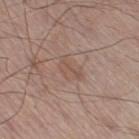follow-up: no biopsy performed (imaged during a skin exam) | anatomic site: the right thigh | patient: male, aged around 55 | image: ~15 mm tile from a whole-body skin photo | tile lighting: white-light | size: about 3.5 mm | automated metrics: a lesion color around L≈52 a*≈17 b*≈25 in CIELAB, roughly 6 lightness units darker than nearby skin, and a normalized border contrast of about 4.5; a border-irregularity rating of about 4.5/10, internal color variation of about 1.5 on a 0–10 scale, and peripheral color asymmetry of about 0.5; a classifier nevus-likeness of about 0/100 and lesion-presence confidence of about 100/100.